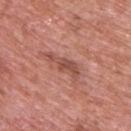image-analysis metrics: a footprint of about 5.5 mm² and an eccentricity of roughly 0.95; an average lesion color of about L≈50 a*≈26 b*≈27 (CIELAB), a lesion–skin lightness drop of about 10, and a normalized border contrast of about 7; a border-irregularity index near 5/10, a within-lesion color-variation index near 1.5/10, and peripheral color asymmetry of about 0.5; a nevus-likeness score of about 0/100
site: the upper back
lesion diameter: ~4.5 mm (longest diameter)
imaging modality: 15 mm crop, total-body photography
illumination: white-light illumination
patient: male, approximately 70 years of age